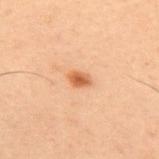Imaged during a routine full-body skin examination; the lesion was not biopsied and no histopathology is available. Located on the upper back. Captured under cross-polarized illumination. A male subject, aged around 55. Cropped from a total-body skin-imaging series; the visible field is about 15 mm. Measured at roughly 2.5 mm in maximum diameter.